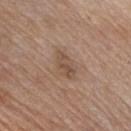Part of a total-body skin-imaging series; this lesion was reviewed on a skin check and was not flagged for biopsy.
This is a white-light tile.
From the chest.
Cropped from a whole-body photographic skin survey; the tile spans about 15 mm.
A male subject in their mid-50s.